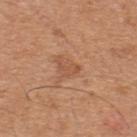Q: Was this lesion biopsied?
A: catalogued during a skin exam; not biopsied
Q: Who is the patient?
A: male, roughly 45 years of age
Q: Automated lesion metrics?
A: an area of roughly 3 mm², a shape eccentricity near 0.8, and a shape-asymmetry score of about 0.55 (0 = symmetric)
Q: How large is the lesion?
A: ~2.5 mm (longest diameter)
Q: What kind of image is this?
A: 15 mm crop, total-body photography
Q: Lesion location?
A: the back
Q: How was the tile lit?
A: white-light illumination